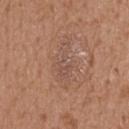Recorded during total-body skin imaging; not selected for excision or biopsy. A female patient, aged around 70. Automated image analysis of the tile measured a lesion–skin lightness drop of about 5 and a normalized lesion–skin contrast near 4.5. And it measured lesion-presence confidence of about 100/100. The lesion is located on the back. A region of skin cropped from a whole-body photographic capture, roughly 15 mm wide. Captured under white-light illumination. About 5 mm across.